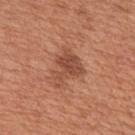A lesion tile, about 15 mm wide, cut from a 3D total-body photograph.
A male patient, aged 63 to 67.
The lesion is located on the upper back.
About 4.5 mm across.
Imaged with white-light lighting.